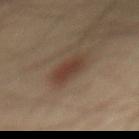{
  "biopsy_status": "not biopsied; imaged during a skin examination",
  "site": "mid back",
  "image": {
    "source": "total-body photography crop",
    "field_of_view_mm": 15
  },
  "patient": {
    "sex": "male",
    "age_approx": 60
  }
}